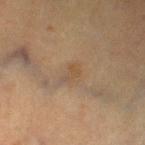follow-up: imaged on a skin check; not biopsied | imaging modality: ~15 mm crop, total-body skin-cancer survey | location: the left thigh | subject: female, aged 53–57.